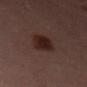The lesion was tiled from a total-body skin photograph and was not biopsied. About 4 mm across. Located on the right thigh. An algorithmic analysis of the crop reported a lesion color around L≈21 a*≈17 b*≈18 in CIELAB, a lesion–skin lightness drop of about 9, and a normalized lesion–skin contrast near 11. It also reported border irregularity of about 2.5 on a 0–10 scale and a peripheral color-asymmetry measure near 1. It also reported a nevus-likeness score of about 95/100 and lesion-presence confidence of about 100/100. This image is a 15 mm lesion crop taken from a total-body photograph. A female patient aged 48 to 52.